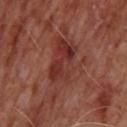No biopsy was performed on this lesion — it was imaged during a full skin examination and was not determined to be concerning. This is a cross-polarized tile. From the upper back. The lesion-visualizer software estimated an automated nevus-likeness rating near 0 out of 100 and a lesion-detection confidence of about 65/100. The subject is a male in their mid- to late 60s. About 9.5 mm across. Cropped from a total-body skin-imaging series; the visible field is about 15 mm.